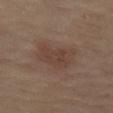notes — total-body-photography surveillance lesion; no biopsy
image source — total-body-photography crop, ~15 mm field of view
lesion diameter — ≈4.5 mm
patient — male, aged around 65
anatomic site — the left thigh
automated metrics — an automated nevus-likeness rating near 5 out of 100 and lesion-presence confidence of about 100/100